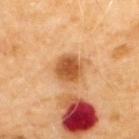Findings:
- workup — imaged on a skin check; not biopsied
- patient — male, aged 68 to 72
- imaging modality — total-body-photography crop, ~15 mm field of view
- body site — the back